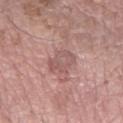subject: male, aged 53 to 57 | image-analysis metrics: a lesion color around L≈55 a*≈21 b*≈22 in CIELAB, about 8 CIELAB-L* units darker than the surrounding skin, and a lesion-to-skin contrast of about 5.5 (normalized; higher = more distinct); a nevus-likeness score of about 0/100 | imaging modality: total-body-photography crop, ~15 mm field of view | body site: the right forearm | lesion size: ~4 mm (longest diameter).